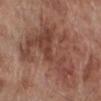Imaged during a routine full-body skin examination; the lesion was not biopsied and no histopathology is available.
This image is a 15 mm lesion crop taken from a total-body photograph.
The lesion's longest dimension is about 11 mm.
A male patient aged 68–72.
On the leg.
The lesion-visualizer software estimated an eccentricity of roughly 0.85 and two-axis asymmetry of about 0.6. The analysis additionally found a border-irregularity index near 9.5/10 and internal color variation of about 6 on a 0–10 scale. And it measured an automated nevus-likeness rating near 5 out of 100 and lesion-presence confidence of about 100/100.